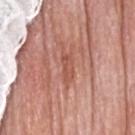Captured during whole-body skin photography for melanoma surveillance; the lesion was not biopsied. Cropped from a total-body skin-imaging series; the visible field is about 15 mm. This is a white-light tile. A female subject about 75 years old. The lesion is located on the head or neck. Longest diameter approximately 3 mm. The lesion-visualizer software estimated a lesion color around L≈53 a*≈27 b*≈30 in CIELAB and a normalized border contrast of about 5.5. And it measured a classifier nevus-likeness of about 0/100 and lesion-presence confidence of about 75/100.